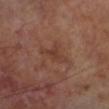Imaged during a routine full-body skin examination; the lesion was not biopsied and no histopathology is available.
A male patient, in their 70s.
Imaged with cross-polarized lighting.
The lesion's longest dimension is about 3.5 mm.
Automated tile analysis of the lesion measured a mean CIELAB color near L≈37 a*≈20 b*≈26, about 6 CIELAB-L* units darker than the surrounding skin, and a lesion-to-skin contrast of about 5.5 (normalized; higher = more distinct). The analysis additionally found a classifier nevus-likeness of about 0/100 and lesion-presence confidence of about 100/100.
A 15 mm crop from a total-body photograph taken for skin-cancer surveillance.
From the left lower leg.